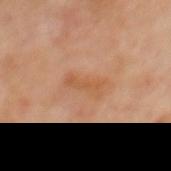Imaged during a routine full-body skin examination; the lesion was not biopsied and no histopathology is available. Measured at roughly 4 mm in maximum diameter. On the mid back. A 15 mm close-up extracted from a 3D total-body photography capture. A male subject, in their 70s. Captured under cross-polarized illumination.